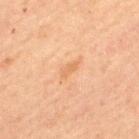Findings:
• workup · imaged on a skin check; not biopsied
• image source · total-body-photography crop, ~15 mm field of view
• location · the upper back
• patient · male, roughly 60 years of age